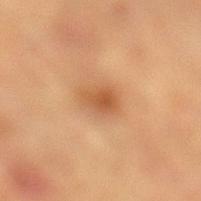{"biopsy_status": "not biopsied; imaged during a skin examination", "lighting": "cross-polarized", "image": {"source": "total-body photography crop", "field_of_view_mm": 15}, "patient": {"sex": "male", "age_approx": "85+"}, "lesion_size": {"long_diameter_mm_approx": 3.5}, "site": "left lower leg", "automated_metrics": {"area_mm2_approx": 6.5, "shape_asymmetry": 0.25, "cielab_L": 49, "cielab_a": 21, "cielab_b": 34, "vs_skin_darker_L": 9.0, "vs_skin_contrast_norm": 6.5}}